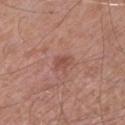notes: catalogued during a skin exam; not biopsied | lesion diameter: ≈2.5 mm | imaging modality: total-body-photography crop, ~15 mm field of view | anatomic site: the left lower leg | lighting: white-light | patient: male, aged 73 to 77 | TBP lesion metrics: a lesion area of about 3.5 mm², an outline eccentricity of about 0.7 (0 = round, 1 = elongated), and a shape-asymmetry score of about 0.3 (0 = symmetric); an average lesion color of about L≈50 a*≈24 b*≈26 (CIELAB), about 7 CIELAB-L* units darker than the surrounding skin, and a normalized lesion–skin contrast near 5.5; an automated nevus-likeness rating near 0 out of 100 and a detector confidence of about 100 out of 100 that the crop contains a lesion.